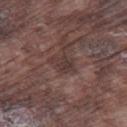The lesion was photographed on a routine skin check and not biopsied; there is no pathology result. Cropped from a whole-body photographic skin survey; the tile spans about 15 mm. The tile uses white-light illumination. The lesion is on the right thigh. The lesion's longest dimension is about 2.5 mm. The subject is a male in their mid-70s.